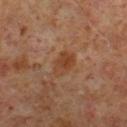follow-up: no biopsy performed (imaged during a skin exam) | lesion diameter: about 3 mm | subject: male, about 60 years old | tile lighting: cross-polarized | imaging modality: total-body-photography crop, ~15 mm field of view | anatomic site: the leg.